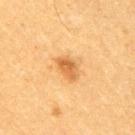biopsy_status: not biopsied; imaged during a skin examination
lighting: cross-polarized
lesion_size:
  long_diameter_mm_approx: 3.0
patient:
  sex: male
  age_approx: 50
automated_metrics:
  area_mm2_approx: 5.0
  eccentricity: 0.8
  shape_asymmetry: 0.25
  cielab_L: 51
  cielab_a: 21
  cielab_b: 39
  vs_skin_contrast_norm: 7.5
  color_variation_0_10: 3.5
  peripheral_color_asymmetry: 1.0
site: right upper arm
image:
  source: total-body photography crop
  field_of_view_mm: 15